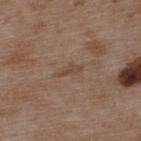No biopsy was performed on this lesion — it was imaged during a full skin examination and was not determined to be concerning. Imaged with white-light lighting. This image is a 15 mm lesion crop taken from a total-body photograph. The lesion is located on the upper back. A male patient aged around 50.